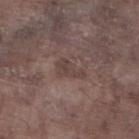Impression: This lesion was catalogued during total-body skin photography and was not selected for biopsy. Background: A lesion tile, about 15 mm wide, cut from a 3D total-body photograph. A male patient approximately 75 years of age. Located on the right lower leg. The tile uses white-light illumination. Longest diameter approximately 3.5 mm.